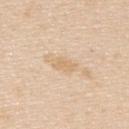Assessment:
Captured during whole-body skin photography for melanoma surveillance; the lesion was not biopsied.
Acquisition and patient details:
On the upper back. Captured under white-light illumination. About 3 mm across. A 15 mm close-up extracted from a 3D total-body photography capture. A male patient aged approximately 45.